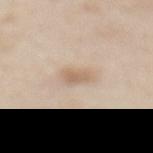Captured during whole-body skin photography for melanoma surveillance; the lesion was not biopsied. On the mid back. The patient is a female roughly 50 years of age. A lesion tile, about 15 mm wide, cut from a 3D total-body photograph.